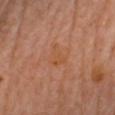No biopsy was performed on this lesion — it was imaged during a full skin examination and was not determined to be concerning. This is a cross-polarized tile. An algorithmic analysis of the crop reported a mean CIELAB color near L≈52 a*≈24 b*≈37, a lesion–skin lightness drop of about 4, and a normalized lesion–skin contrast near 6. The analysis additionally found internal color variation of about 1 on a 0–10 scale and radial color variation of about 0. The software also gave lesion-presence confidence of about 100/100. A female patient in their 60s. Located on the chest. Cropped from a whole-body photographic skin survey; the tile spans about 15 mm. The lesion's longest dimension is about 3 mm.